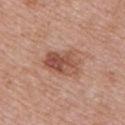Q: Where on the body is the lesion?
A: the chest
Q: How was this image acquired?
A: total-body-photography crop, ~15 mm field of view
Q: Who is the patient?
A: female, aged around 50